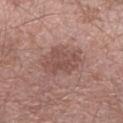biopsy_status: not biopsied; imaged during a skin examination
lesion_size:
  long_diameter_mm_approx: 5.0
patient:
  sex: male
  age_approx: 55
image:
  source: total-body photography crop
  field_of_view_mm: 15
site: arm
automated_metrics:
  cielab_L: 50
  cielab_a: 20
  cielab_b: 23
  vs_skin_darker_L: 8.0
  vs_skin_contrast_norm: 6.0
  color_variation_0_10: 3.0
  peripheral_color_asymmetry: 1.0
  nevus_likeness_0_100: 0
  lesion_detection_confidence_0_100: 100
lighting: white-light